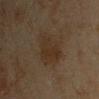notes: catalogued during a skin exam; not biopsied | tile lighting: cross-polarized | image-analysis metrics: a mean CIELAB color near L≈24 a*≈10 b*≈21, about 4 CIELAB-L* units darker than the surrounding skin, and a lesion-to-skin contrast of about 6.5 (normalized; higher = more distinct); a border-irregularity index near 3/10 and a color-variation rating of about 3/10 | patient: male, approximately 45 years of age | body site: the arm | image source: ~15 mm tile from a whole-body skin photo.